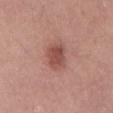Notes:
* follow-up: total-body-photography surveillance lesion; no biopsy
* site: the abdomen
* image: ~15 mm tile from a whole-body skin photo
* illumination: white-light
* size: about 3.5 mm
* patient: male, aged around 60